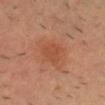| feature | finding |
|---|---|
| patient | male, aged around 35 |
| anatomic site | the head or neck |
| illumination | cross-polarized illumination |
| acquisition | total-body-photography crop, ~15 mm field of view |